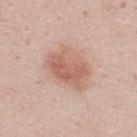Recorded during total-body skin imaging; not selected for excision or biopsy. On the upper back. A 15 mm crop from a total-body photograph taken for skin-cancer surveillance. A male subject, aged 23–27.